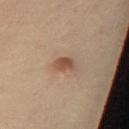| key | value |
|---|---|
| follow-up | imaged on a skin check; not biopsied |
| body site | the chest |
| subject | female, aged around 40 |
| image source | 15 mm crop, total-body photography |
| lesion diameter | ≈2.5 mm |